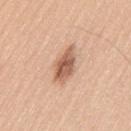biopsy status: no biopsy performed (imaged during a skin exam)
diameter: ~4.5 mm (longest diameter)
imaging modality: total-body-photography crop, ~15 mm field of view
illumination: white-light illumination
location: the left thigh
automated metrics: an area of roughly 7.5 mm² and an outline eccentricity of about 0.85 (0 = round, 1 = elongated); a border-irregularity rating of about 2.5/10 and internal color variation of about 5 on a 0–10 scale; a nevus-likeness score of about 70/100
subject: male, approximately 40 years of age